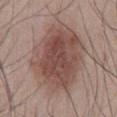Findings:
• illumination · white-light illumination
• anatomic site · the mid back
• subject · male, in their mid- to late 40s
• TBP lesion metrics · an outline eccentricity of about 0.65 (0 = round, 1 = elongated); a border-irregularity rating of about 2/10, a within-lesion color-variation index near 5/10, and a peripheral color-asymmetry measure near 1.5; a nevus-likeness score of about 80/100 and a detector confidence of about 100 out of 100 that the crop contains a lesion
• acquisition · 15 mm crop, total-body photography
• diameter · ~8.5 mm (longest diameter)